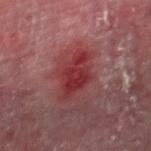notes = imaged on a skin check; not biopsied
acquisition = ~15 mm crop, total-body skin-cancer survey
location = the leg
lesion diameter = ≈5 mm
illumination = cross-polarized illumination
subject = male, aged 53–57